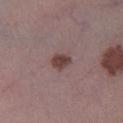Q: Is there a histopathology result?
A: total-body-photography surveillance lesion; no biopsy
Q: Patient demographics?
A: male, aged around 60
Q: What is the anatomic site?
A: the right lower leg
Q: What kind of image is this?
A: total-body-photography crop, ~15 mm field of view